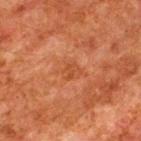No biopsy was performed on this lesion — it was imaged during a full skin examination and was not determined to be concerning.